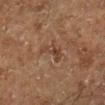{"biopsy_status": "not biopsied; imaged during a skin examination", "site": "leg", "image": {"source": "total-body photography crop", "field_of_view_mm": 15}, "automated_metrics": {"area_mm2_approx": 4.0, "eccentricity": 0.9, "border_irregularity_0_10": 6.5, "color_variation_0_10": 0.0, "peripheral_color_asymmetry": 0.0}, "lesion_size": {"long_diameter_mm_approx": 3.5}, "patient": {"sex": "male", "age_approx": 75}}